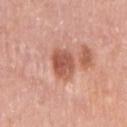The lesion was tiled from a total-body skin photograph and was not biopsied. About 4 mm across. A female subject, aged 38–42. The lesion is located on the right upper arm. A 15 mm crop from a total-body photograph taken for skin-cancer surveillance.